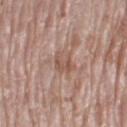Image and clinical context: A roughly 15 mm field-of-view crop from a total-body skin photograph. On the left thigh. Automated image analysis of the tile measured a footprint of about 5 mm², an eccentricity of roughly 0.8, and two-axis asymmetry of about 0.3. The analysis additionally found a lesion color around L≈54 a*≈19 b*≈26 in CIELAB and a lesion–skin lightness drop of about 8. It also reported a classifier nevus-likeness of about 0/100 and a lesion-detection confidence of about 85/100. The subject is a female aged around 75. Longest diameter approximately 3.5 mm.